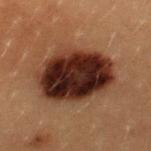The lesion is on the back. Imaged with cross-polarized lighting. A roughly 15 mm field-of-view crop from a total-body skin photograph. The subject is a female about 20 years old.